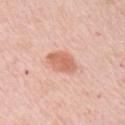Q: Is there a histopathology result?
A: imaged on a skin check; not biopsied
Q: What are the patient's age and sex?
A: female, aged around 65
Q: What is the imaging modality?
A: ~15 mm tile from a whole-body skin photo
Q: Where on the body is the lesion?
A: the right upper arm